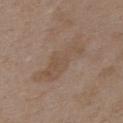{
  "biopsy_status": "not biopsied; imaged during a skin examination",
  "lighting": "white-light",
  "image": {
    "source": "total-body photography crop",
    "field_of_view_mm": 15
  },
  "patient": {
    "sex": "female",
    "age_approx": 35
  },
  "automated_metrics": {
    "color_variation_0_10": 2.5,
    "peripheral_color_asymmetry": 0.5,
    "nevus_likeness_0_100": 0,
    "lesion_detection_confidence_0_100": 100
  },
  "site": "upper back"
}